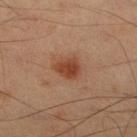Assessment:
The lesion was photographed on a routine skin check and not biopsied; there is no pathology result.
Context:
This image is a 15 mm lesion crop taken from a total-body photograph. Located on the leg. The patient is a male approximately 60 years of age. Imaged with cross-polarized lighting. Approximately 3 mm at its widest. An algorithmic analysis of the crop reported a lesion area of about 6 mm², an eccentricity of roughly 0.6, and a symmetry-axis asymmetry near 0.25. And it measured a mean CIELAB color near L≈38 a*≈22 b*≈29, roughly 10 lightness units darker than nearby skin, and a lesion-to-skin contrast of about 8.5 (normalized; higher = more distinct). And it measured a border-irregularity index near 2.5/10 and a color-variation rating of about 2.5/10. And it measured a nevus-likeness score of about 100/100 and a lesion-detection confidence of about 100/100.